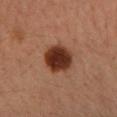biopsy_status: not biopsied; imaged during a skin examination
lesion_size:
  long_diameter_mm_approx: 4.0
patient:
  sex: female
  age_approx: 40
image:
  source: total-body photography crop
  field_of_view_mm: 15
site: arm
automated_metrics:
  cielab_L: 35
  cielab_a: 24
  cielab_b: 30
  vs_skin_contrast_norm: 14.0
  nevus_likeness_0_100: 100